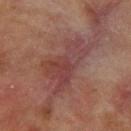biopsy status = total-body-photography surveillance lesion; no biopsy | lesion size = about 7.5 mm | image = total-body-photography crop, ~15 mm field of view | location = the left forearm | patient = female, aged 78 to 82.